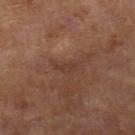workup=imaged on a skin check; not biopsied | image-analysis metrics=a border-irregularity index near 4/10 and internal color variation of about 0 on a 0–10 scale; lesion-presence confidence of about 55/100 | illumination=cross-polarized | patient=female, about 60 years old | location=the right lower leg | image=total-body-photography crop, ~15 mm field of view.